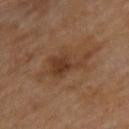{
  "biopsy_status": "not biopsied; imaged during a skin examination",
  "lighting": "cross-polarized",
  "patient": {
    "sex": "male",
    "age_approx": 70
  },
  "site": "upper back",
  "image": {
    "source": "total-body photography crop",
    "field_of_view_mm": 15
  },
  "lesion_size": {
    "long_diameter_mm_approx": 5.5
  }
}